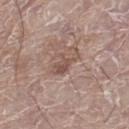workup = catalogued during a skin exam; not biopsied
body site = the left leg
patient = male, aged approximately 80
acquisition = total-body-photography crop, ~15 mm field of view